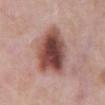Notes:
• notes · no biopsy performed (imaged during a skin exam)
• acquisition · ~15 mm crop, total-body skin-cancer survey
• automated lesion analysis · a border-irregularity rating of about 2.5/10, internal color variation of about 8 on a 0–10 scale, and a peripheral color-asymmetry measure near 2; a nevus-likeness score of about 85/100 and a lesion-detection confidence of about 100/100
• anatomic site · the abdomen
• subject · male, aged 53–57